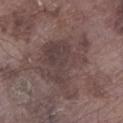Q: Was a biopsy performed?
A: total-body-photography surveillance lesion; no biopsy
Q: What is the anatomic site?
A: the left lower leg
Q: What did automated image analysis measure?
A: a footprint of about 27 mm², an outline eccentricity of about 0.55 (0 = round, 1 = elongated), and a shape-asymmetry score of about 0.5 (0 = symmetric)
Q: What kind of image is this?
A: 15 mm crop, total-body photography
Q: Patient demographics?
A: male, aged around 75
Q: What is the lesion's diameter?
A: ≈8 mm
Q: Illumination type?
A: white-light illumination